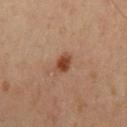Assessment:
This lesion was catalogued during total-body skin photography and was not selected for biopsy.
Acquisition and patient details:
A male patient, in their 40s. The lesion is located on the left upper arm. Approximately 2.5 mm at its widest. Cropped from a total-body skin-imaging series; the visible field is about 15 mm.